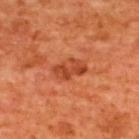Captured during whole-body skin photography for melanoma surveillance; the lesion was not biopsied.